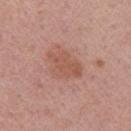Captured during whole-body skin photography for melanoma surveillance; the lesion was not biopsied. A close-up tile cropped from a whole-body skin photograph, about 15 mm across. Automated image analysis of the tile measured a footprint of about 8.5 mm², an outline eccentricity of about 0.75 (0 = round, 1 = elongated), and a shape-asymmetry score of about 0.35 (0 = symmetric). It also reported a mean CIELAB color near L≈54 a*≈24 b*≈28, a lesion–skin lightness drop of about 7, and a normalized lesion–skin contrast near 6. The software also gave a border-irregularity rating of about 4/10, internal color variation of about 2 on a 0–10 scale, and radial color variation of about 0.5. This is a white-light tile. Longest diameter approximately 4.5 mm. The lesion is on the arm. A female patient, aged approximately 50.